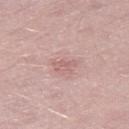  biopsy_status: not biopsied; imaged during a skin examination
  site: leg
  patient:
    sex: male
    age_approx: 50
  image:
    source: total-body photography crop
    field_of_view_mm: 15